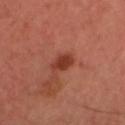  automated_metrics:
    eccentricity: 0.7
    shape_asymmetry: 0.15
    cielab_L: 37
    cielab_a: 29
    cielab_b: 30
    vs_skin_darker_L: 11.0
    vs_skin_contrast_norm: 9.0
  patient:
    sex: male
    age_approx: 35
  site: head or neck
  image:
    source: total-body photography crop
    field_of_view_mm: 15
  lesion_size:
    long_diameter_mm_approx: 3.0